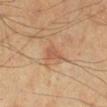- workup · total-body-photography surveillance lesion; no biopsy
- image · total-body-photography crop, ~15 mm field of view
- patient · male, aged around 70
- body site · the leg
- size · ≈3 mm
- image-analysis metrics · a shape eccentricity near 0.85 and a shape-asymmetry score of about 0.45 (0 = symmetric); border irregularity of about 4 on a 0–10 scale and a within-lesion color-variation index near 1/10